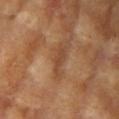follow-up = no biopsy performed (imaged during a skin exam); subject = female, in their mid-70s; image source = ~15 mm crop, total-body skin-cancer survey; anatomic site = the arm.